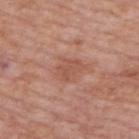Part of a total-body skin-imaging series; this lesion was reviewed on a skin check and was not flagged for biopsy.
The patient is a male aged approximately 75.
A roughly 15 mm field-of-view crop from a total-body skin photograph.
From the upper back.